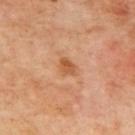{"biopsy_status": "not biopsied; imaged during a skin examination", "image": {"source": "total-body photography crop", "field_of_view_mm": 15}, "patient": {"sex": "male", "age_approx": 70}, "lesion_size": {"long_diameter_mm_approx": 2.5}, "site": "upper back", "automated_metrics": {"border_irregularity_0_10": 2.5, "color_variation_0_10": 3.0, "nevus_likeness_0_100": 35, "lesion_detection_confidence_0_100": 100}, "lighting": "cross-polarized"}